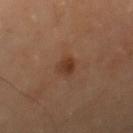Impression: Recorded during total-body skin imaging; not selected for excision or biopsy. Context: The patient is a female roughly 70 years of age. About 2.5 mm across. Automated image analysis of the tile measured an outline eccentricity of about 0.5 (0 = round, 1 = elongated) and a shape-asymmetry score of about 0.25 (0 = symmetric). It also reported a mean CIELAB color near L≈37 a*≈21 b*≈30 and a normalized border contrast of about 7.5. And it measured a border-irregularity index near 2/10, a within-lesion color-variation index near 3/10, and radial color variation of about 1. And it measured an automated nevus-likeness rating near 85 out of 100. Cropped from a whole-body photographic skin survey; the tile spans about 15 mm. On the right thigh.